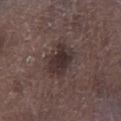Impression: No biopsy was performed on this lesion — it was imaged during a full skin examination and was not determined to be concerning. Background: Measured at roughly 4 mm in maximum diameter. A male subject, roughly 70 years of age. Captured under white-light illumination. The lesion is on the left lower leg. Cropped from a whole-body photographic skin survey; the tile spans about 15 mm.